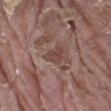Captured during whole-body skin photography for melanoma surveillance; the lesion was not biopsied. The subject is a male roughly 40 years of age. Longest diameter approximately 3 mm. Cropped from a total-body skin-imaging series; the visible field is about 15 mm. From the lower back. This is a white-light tile.